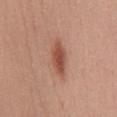Q: Was this lesion biopsied?
A: no biopsy performed (imaged during a skin exam)
Q: Who is the patient?
A: female, roughly 25 years of age
Q: How was the tile lit?
A: white-light
Q: Where on the body is the lesion?
A: the mid back
Q: What did automated image analysis measure?
A: border irregularity of about 2.5 on a 0–10 scale, a color-variation rating of about 3/10, and a peripheral color-asymmetry measure near 1
Q: What kind of image is this?
A: ~15 mm crop, total-body skin-cancer survey